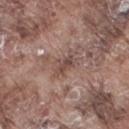Clinical impression:
No biopsy was performed on this lesion — it was imaged during a full skin examination and was not determined to be concerning.